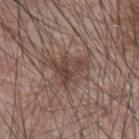notes — no biopsy performed (imaged during a skin exam) | patient — male, aged 73 to 77 | location — the left forearm | acquisition — ~15 mm tile from a whole-body skin photo.